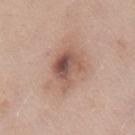Background: The lesion is located on the chest. The lesion's longest dimension is about 4.5 mm. Imaged with white-light lighting. A region of skin cropped from a whole-body photographic capture, roughly 15 mm wide. The subject is a male about 55 years old. Automated tile analysis of the lesion measured roughly 13 lightness units darker than nearby skin. The analysis additionally found an automated nevus-likeness rating near 5 out of 100.